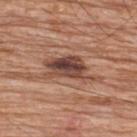notes = total-body-photography surveillance lesion; no biopsy
patient = male, in their 80s
tile lighting = white-light
image source = ~15 mm tile from a whole-body skin photo
lesion diameter = ~6.5 mm (longest diameter)
site = the upper back